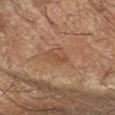Q: Was this lesion biopsied?
A: no biopsy performed (imaged during a skin exam)
Q: What are the patient's age and sex?
A: male, in their mid-60s
Q: What is the imaging modality?
A: total-body-photography crop, ~15 mm field of view
Q: Illumination type?
A: cross-polarized illumination
Q: How large is the lesion?
A: ≈2.5 mm
Q: Lesion location?
A: the right forearm